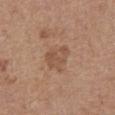The lesion was tiled from a total-body skin photograph and was not biopsied. Cropped from a total-body skin-imaging series; the visible field is about 15 mm. A female patient in their mid-60s. From the abdomen. Captured under white-light illumination. About 3.5 mm across.